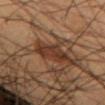Assessment: Imaged during a routine full-body skin examination; the lesion was not biopsied and no histopathology is available. Background: The total-body-photography lesion software estimated an eccentricity of roughly 0.9 and a symmetry-axis asymmetry near 0.25. Cropped from a whole-body photographic skin survey; the tile spans about 15 mm. The tile uses cross-polarized illumination. A male subject, aged around 55. The lesion's longest dimension is about 5 mm. Located on the left forearm.